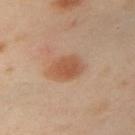| key | value |
|---|---|
| biopsy status | no biopsy performed (imaged during a skin exam) |
| subject | female, aged 38–42 |
| lesion diameter | ~4 mm (longest diameter) |
| imaging modality | 15 mm crop, total-body photography |
| automated lesion analysis | a footprint of about 9 mm² and an outline eccentricity of about 0.75 (0 = round, 1 = elongated); a color-variation rating of about 3/10 and radial color variation of about 1 |
| body site | the left upper arm |